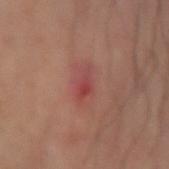Captured during whole-body skin photography for melanoma surveillance; the lesion was not biopsied. An algorithmic analysis of the crop reported a within-lesion color-variation index near 2/10 and peripheral color asymmetry of about 0.5. The lesion is on the left forearm. Measured at roughly 3 mm in maximum diameter. A 15 mm close-up extracted from a 3D total-body photography capture.